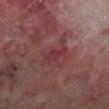No biopsy was performed on this lesion — it was imaged during a full skin examination and was not determined to be concerning.
Imaged with cross-polarized lighting.
The lesion is on the right lower leg.
About 3 mm across.
The patient is a male roughly 70 years of age.
A 15 mm close-up extracted from a 3D total-body photography capture.